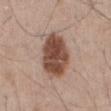Impression: Recorded during total-body skin imaging; not selected for excision or biopsy. Clinical summary: Located on the mid back. A 15 mm close-up extracted from a 3D total-body photography capture. Measured at roughly 6 mm in maximum diameter. The patient is a male in their 40s. Automated image analysis of the tile measured a lesion–skin lightness drop of about 16 and a normalized border contrast of about 11.5. And it measured an automated nevus-likeness rating near 95 out of 100 and a lesion-detection confidence of about 100/100.